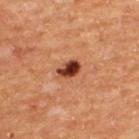Imaged during a routine full-body skin examination; the lesion was not biopsied and no histopathology is available. The lesion is on the back. A male subject aged approximately 65. Approximately 3 mm at its widest. A 15 mm close-up extracted from a 3D total-body photography capture. Captured under cross-polarized illumination.